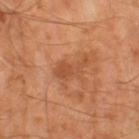Recorded during total-body skin imaging; not selected for excision or biopsy. A male patient, in their mid-60s. Approximately 4 mm at its widest. Imaged with cross-polarized lighting. An algorithmic analysis of the crop reported roughly 8 lightness units darker than nearby skin and a normalized border contrast of about 5.5. The software also gave an automated nevus-likeness rating near 5 out of 100 and a detector confidence of about 100 out of 100 that the crop contains a lesion. A 15 mm close-up extracted from a 3D total-body photography capture.